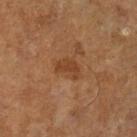A 15 mm close-up tile from a total-body photography series done for melanoma screening.
The lesion is on the left lower leg.
Captured under cross-polarized illumination.
Measured at roughly 3 mm in maximum diameter.
A male patient, approximately 65 years of age.
Automated image analysis of the tile measured internal color variation of about 2.5 on a 0–10 scale and peripheral color asymmetry of about 0.5.